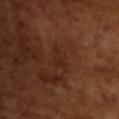Q: Was this lesion biopsied?
A: total-body-photography surveillance lesion; no biopsy
Q: Who is the patient?
A: male, in their mid-60s
Q: How was the tile lit?
A: cross-polarized
Q: What did automated image analysis measure?
A: a classifier nevus-likeness of about 0/100
Q: What kind of image is this?
A: ~15 mm crop, total-body skin-cancer survey
Q: How large is the lesion?
A: about 2.5 mm